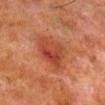The lesion is on the right lower leg. A male patient, approximately 80 years of age. The recorded lesion diameter is about 5 mm. Cropped from a total-body skin-imaging series; the visible field is about 15 mm.